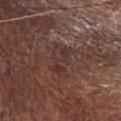Clinical summary: The lesion is located on the right forearm. Automated tile analysis of the lesion measured border irregularity of about 3.5 on a 0–10 scale, a within-lesion color-variation index near 3.5/10, and peripheral color asymmetry of about 1.5. A 15 mm close-up tile from a total-body photography series done for melanoma screening. The subject is a male aged approximately 70.